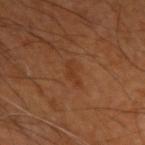notes — total-body-photography surveillance lesion; no biopsy | lesion size — about 3 mm | image source — ~15 mm tile from a whole-body skin photo | patient — male, in their 60s | site — the left upper arm.